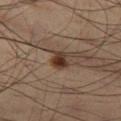  biopsy_status: not biopsied; imaged during a skin examination
  lighting: cross-polarized
  image:
    source: total-body photography crop
    field_of_view_mm: 15
  automated_metrics:
    area_mm2_approx: 4.0
    eccentricity: 0.6
    cielab_L: 33
    cielab_a: 17
    cielab_b: 25
    vs_skin_darker_L: 13.0
    vs_skin_contrast_norm: 11.5
    border_irregularity_0_10: 3.0
    peripheral_color_asymmetry: 1.5
    nevus_likeness_0_100: 95
    lesion_detection_confidence_0_100: 100
  site: left thigh
  patient:
    sex: male
    age_approx: 65